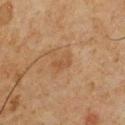biopsy status: imaged on a skin check; not biopsied
lighting: cross-polarized
subject: male, roughly 60 years of age
lesion size: ~2.5 mm (longest diameter)
site: the chest
acquisition: ~15 mm crop, total-body skin-cancer survey